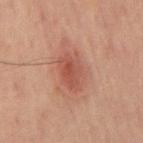notes=no biopsy performed (imaged during a skin exam) | lighting=cross-polarized | image source=total-body-photography crop, ~15 mm field of view | size=~4.5 mm (longest diameter) | automated metrics=a lesion color around L≈42 a*≈22 b*≈24 in CIELAB, roughly 8 lightness units darker than nearby skin, and a normalized border contrast of about 6.5; a nevus-likeness score of about 55/100 and a lesion-detection confidence of about 100/100 | anatomic site=the chest | subject=male, aged around 70.